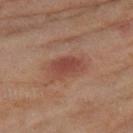Assessment: Imaged during a routine full-body skin examination; the lesion was not biopsied and no histopathology is available. Background: A 15 mm close-up tile from a total-body photography series done for melanoma screening. The lesion is on the right thigh. The subject is a female aged around 60.